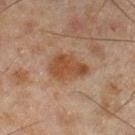Assessment: Imaged during a routine full-body skin examination; the lesion was not biopsied and no histopathology is available. Context: The total-body-photography lesion software estimated a normalized border contrast of about 8. The software also gave a lesion-detection confidence of about 100/100. A male patient, aged approximately 45. The lesion is on the right lower leg. A 15 mm crop from a total-body photograph taken for skin-cancer surveillance.